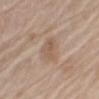site: the left upper arm
automated lesion analysis: an outline eccentricity of about 0.6 (0 = round, 1 = elongated) and a symmetry-axis asymmetry near 0.4; about 7 CIELAB-L* units darker than the surrounding skin and a normalized lesion–skin contrast near 5; a color-variation rating of about 2.5/10 and radial color variation of about 1; a nevus-likeness score of about 0/100
imaging modality: total-body-photography crop, ~15 mm field of view
lesion size: ~3 mm (longest diameter)
tile lighting: white-light illumination
patient: male, approximately 65 years of age